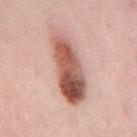notes=total-body-photography surveillance lesion; no biopsy
image source=~15 mm crop, total-body skin-cancer survey
body site=the mid back
patient=female, in their 40s